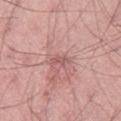biopsy status: no biopsy performed (imaged during a skin exam) | patient: male, aged 43 to 47 | location: the left thigh | image: total-body-photography crop, ~15 mm field of view.